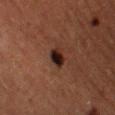| key | value |
|---|---|
| biopsy status | no biopsy performed (imaged during a skin exam) |